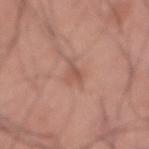Q: Was a biopsy performed?
A: no biopsy performed (imaged during a skin exam)
Q: Who is the patient?
A: male, aged 53–57
Q: What did automated image analysis measure?
A: a lesion area of about 3 mm², an outline eccentricity of about 0.85 (0 = round, 1 = elongated), and a symmetry-axis asymmetry near 0.55; a lesion color around L≈54 a*≈23 b*≈28 in CIELAB
Q: What is the imaging modality?
A: ~15 mm tile from a whole-body skin photo
Q: Lesion size?
A: about 3 mm
Q: What is the anatomic site?
A: the front of the torso